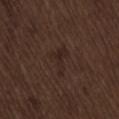| field | value |
|---|---|
| biopsy status | imaged on a skin check; not biopsied |
| subject | male, roughly 70 years of age |
| illumination | white-light |
| image | ~15 mm crop, total-body skin-cancer survey |
| location | the lower back |
| automated metrics | a border-irregularity rating of about 6/10, a within-lesion color-variation index near 2.5/10, and radial color variation of about 1; a nevus-likeness score of about 5/100 and a lesion-detection confidence of about 100/100 |